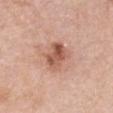  site: chest
  image:
    source: total-body photography crop
    field_of_view_mm: 15
  patient:
    sex: female
    age_approx: 60
  automated_metrics:
    area_mm2_approx: 9.5
    shape_asymmetry: 0.2
    vs_skin_darker_L: 12.0
    vs_skin_contrast_norm: 8.0
    border_irregularity_0_10: 2.5
    color_variation_0_10: 7.0
  lesion_size:
    long_diameter_mm_approx: 4.0
  lighting: white-light